A male patient, aged 43–47. Located on the leg. A 15 mm close-up extracted from a 3D total-body photography capture. Imaged with white-light lighting. Measured at roughly 3 mm in maximum diameter.
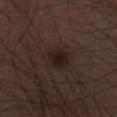Histopathology of the biopsied lesion showed an indeterminate (borderline) lesion: atypical melanocytic neoplasm.